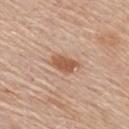Clinical impression:
Captured during whole-body skin photography for melanoma surveillance; the lesion was not biopsied.
Clinical summary:
Imaged with white-light lighting. The patient is a female aged around 65. Automated tile analysis of the lesion measured an area of roughly 5.5 mm², an eccentricity of roughly 0.8, and a symmetry-axis asymmetry near 0.25. A region of skin cropped from a whole-body photographic capture, roughly 15 mm wide. The lesion's longest dimension is about 3.5 mm. The lesion is on the upper back.